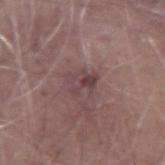  biopsy_status: not biopsied; imaged during a skin examination
  image:
    source: total-body photography crop
    field_of_view_mm: 15
  patient:
    sex: male
    age_approx: 65
  lighting: white-light
  lesion_size:
    long_diameter_mm_approx: 3.0
  site: right forearm
  automated_metrics:
    cielab_L: 42
    cielab_a: 19
    cielab_b: 16
    vs_skin_contrast_norm: 6.5
    nevus_likeness_0_100: 0
    lesion_detection_confidence_0_100: 90